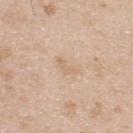follow-up = total-body-photography surveillance lesion; no biopsy | tile lighting = white-light illumination | subject = male, about 25 years old | image source = ~15 mm crop, total-body skin-cancer survey | location = the back.